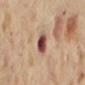The lesion was tiled from a total-body skin photograph and was not biopsied.
Measured at roughly 2.5 mm in maximum diameter.
A 15 mm close-up extracted from a 3D total-body photography capture.
On the mid back.
Automated image analysis of the tile measured radial color variation of about 3. And it measured an automated nevus-likeness rating near 0 out of 100 and a detector confidence of about 100 out of 100 that the crop contains a lesion.
A female patient, aged 53 to 57.